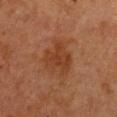Assessment: No biopsy was performed on this lesion — it was imaged during a full skin examination and was not determined to be concerning. Background: The total-body-photography lesion software estimated a shape eccentricity near 0.55. The analysis additionally found an automated nevus-likeness rating near 0 out of 100 and lesion-presence confidence of about 100/100. Imaged with cross-polarized lighting. A female subject, aged 53 to 57. This image is a 15 mm lesion crop taken from a total-body photograph. Located on the chest. Measured at roughly 4 mm in maximum diameter.